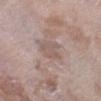workup — imaged on a skin check; not biopsied
location — the left lower leg
tile lighting — white-light
subject — female, aged 68–72
lesion diameter — about 3.5 mm
acquisition — 15 mm crop, total-body photography
automated metrics — a lesion area of about 6.5 mm², a shape eccentricity near 0.6, and two-axis asymmetry of about 0.3; a mean CIELAB color near L≈56 a*≈15 b*≈21, roughly 6 lightness units darker than nearby skin, and a lesion-to-skin contrast of about 4.5 (normalized; higher = more distinct); an automated nevus-likeness rating near 0 out of 100